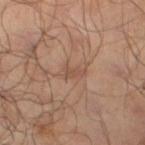Findings:
• notes · total-body-photography surveillance lesion; no biopsy
• anatomic site · the leg
• patient · male, in their mid-60s
• acquisition · total-body-photography crop, ~15 mm field of view